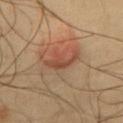{"biopsy_status": "not biopsied; imaged during a skin examination", "lighting": "cross-polarized", "automated_metrics": {"area_mm2_approx": 5.5, "shape_asymmetry": 0.5}, "patient": {"sex": "male", "age_approx": 65}, "lesion_size": {"long_diameter_mm_approx": 4.0}, "site": "chest", "image": {"source": "total-body photography crop", "field_of_view_mm": 15}}